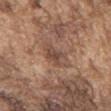Case summary:
• follow-up: catalogued during a skin exam; not biopsied
• patient: male, in their mid- to late 70s
• acquisition: total-body-photography crop, ~15 mm field of view
• anatomic site: the chest
• diameter: ~4 mm (longest diameter)